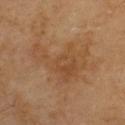– notes — total-body-photography surveillance lesion; no biopsy
– site — the upper back
– tile lighting — cross-polarized
– patient — male, about 70 years old
– lesion diameter — ~7.5 mm (longest diameter)
– acquisition — 15 mm crop, total-body photography